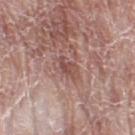Case summary:
– workup: imaged on a skin check; not biopsied
– image: 15 mm crop, total-body photography
– tile lighting: white-light
– anatomic site: the left thigh
– patient: female, approximately 60 years of age